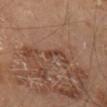Part of a total-body skin-imaging series; this lesion was reviewed on a skin check and was not flagged for biopsy.
The total-body-photography lesion software estimated a lesion–skin lightness drop of about 8. It also reported a nevus-likeness score of about 0/100 and a detector confidence of about 95 out of 100 that the crop contains a lesion.
Captured under cross-polarized illumination.
Located on the right thigh.
A male subject, in their 70s.
Longest diameter approximately 3 mm.
Cropped from a total-body skin-imaging series; the visible field is about 15 mm.